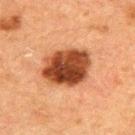Clinical summary: Longest diameter approximately 5.5 mm. Located on the upper back. Cropped from a total-body skin-imaging series; the visible field is about 15 mm. An algorithmic analysis of the crop reported an eccentricity of roughly 0.65 and a symmetry-axis asymmetry near 0.15. A male subject, in their 50s.